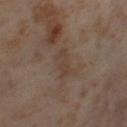Q: Was this lesion biopsied?
A: no biopsy performed (imaged during a skin exam)
Q: Lesion size?
A: about 3.5 mm
Q: What are the patient's age and sex?
A: female, aged 53–57
Q: Automated lesion metrics?
A: a mean CIELAB color near L≈40 a*≈14 b*≈24, roughly 5 lightness units darker than nearby skin, and a lesion-to-skin contrast of about 5 (normalized; higher = more distinct); an automated nevus-likeness rating near 0 out of 100 and a lesion-detection confidence of about 100/100
Q: What kind of image is this?
A: ~15 mm crop, total-body skin-cancer survey
Q: Lesion location?
A: the left thigh